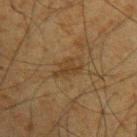Clinical impression: Part of a total-body skin-imaging series; this lesion was reviewed on a skin check and was not flagged for biopsy. Background: A male subject, aged approximately 65. A roughly 15 mm field-of-view crop from a total-body skin photograph. About 3.5 mm across. The lesion is located on the left upper arm. Captured under cross-polarized illumination.